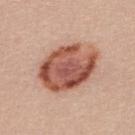Image and clinical context:
The subject is a female aged 23–27. The lesion is on the upper back. Automated tile analysis of the lesion measured an eccentricity of roughly 0.75 and two-axis asymmetry of about 0.15. The software also gave about 16 CIELAB-L* units darker than the surrounding skin and a lesion-to-skin contrast of about 10.5 (normalized; higher = more distinct). And it measured a border-irregularity index near 2/10, internal color variation of about 8.5 on a 0–10 scale, and peripheral color asymmetry of about 3. And it measured a classifier nevus-likeness of about 50/100 and a detector confidence of about 100 out of 100 that the crop contains a lesion. Captured under white-light illumination. A region of skin cropped from a whole-body photographic capture, roughly 15 mm wide. Longest diameter approximately 7.5 mm.